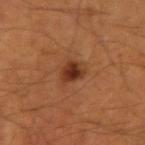Part of a total-body skin-imaging series; this lesion was reviewed on a skin check and was not flagged for biopsy.
A male subject approximately 65 years of age.
The total-body-photography lesion software estimated an area of roughly 4.5 mm², an outline eccentricity of about 0.45 (0 = round, 1 = elongated), and a symmetry-axis asymmetry near 0.25. It also reported a mean CIELAB color near L≈31 a*≈23 b*≈30, about 11 CIELAB-L* units darker than the surrounding skin, and a normalized border contrast of about 10.5.
From the left upper arm.
Longest diameter approximately 2.5 mm.
A 15 mm close-up tile from a total-body photography series done for melanoma screening.